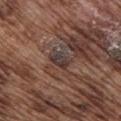Findings:
– biopsy status · catalogued during a skin exam; not biopsied
– body site · the chest
– tile lighting · white-light
– image · ~15 mm crop, total-body skin-cancer survey
– subject · male, aged 73 to 77
– size · about 2.5 mm